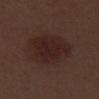follow-up: catalogued during a skin exam; not biopsied | subject: male, approximately 70 years of age | body site: the right thigh | lighting: white-light | acquisition: ~15 mm tile from a whole-body skin photo.